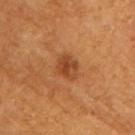Part of a total-body skin-imaging series; this lesion was reviewed on a skin check and was not flagged for biopsy. On the right upper arm. A male patient, aged around 55. A 15 mm close-up extracted from a 3D total-body photography capture.